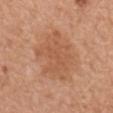No biopsy was performed on this lesion — it was imaged during a full skin examination and was not determined to be concerning.
A 15 mm close-up tile from a total-body photography series done for melanoma screening.
The recorded lesion diameter is about 7 mm.
On the mid back.
The tile uses white-light illumination.
A male subject, aged 68–72.